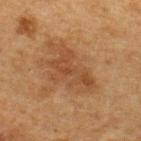biopsy_status: not biopsied; imaged during a skin examination
lighting: cross-polarized
image:
  source: total-body photography crop
  field_of_view_mm: 15
lesion_size:
  long_diameter_mm_approx: 6.0
patient:
  sex: male
  age_approx: 50
site: upper back
automated_metrics:
  eccentricity: 0.8
  shape_asymmetry: 0.55
  border_irregularity_0_10: 8.5
  color_variation_0_10: 2.5